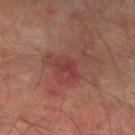Assessment: The lesion was photographed on a routine skin check and not biopsied; there is no pathology result. Clinical summary: Automated tile analysis of the lesion measured a mean CIELAB color near L≈32 a*≈24 b*≈20, a lesion–skin lightness drop of about 5, and a lesion-to-skin contrast of about 5 (normalized; higher = more distinct). The analysis additionally found a border-irregularity rating of about 5/10 and a color-variation rating of about 0.5/10. The lesion is on the left lower leg. A lesion tile, about 15 mm wide, cut from a 3D total-body photograph. Measured at roughly 3 mm in maximum diameter. A male patient about 75 years old.